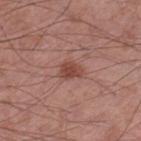The lesion was photographed on a routine skin check and not biopsied; there is no pathology result. Approximately 2.5 mm at its widest. A male subject aged around 55. Cropped from a whole-body photographic skin survey; the tile spans about 15 mm. The lesion-visualizer software estimated a lesion area of about 4.5 mm², an outline eccentricity of about 0.7 (0 = round, 1 = elongated), and two-axis asymmetry of about 0.35. It also reported a lesion color around L≈46 a*≈23 b*≈26 in CIELAB and a lesion-to-skin contrast of about 7.5 (normalized; higher = more distinct). Located on the left thigh.